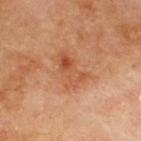Recorded during total-body skin imaging; not selected for excision or biopsy. A 15 mm close-up tile from a total-body photography series done for melanoma screening. The lesion is on the upper back. Longest diameter approximately 4.5 mm. This is a cross-polarized tile. The subject is a male aged approximately 70.